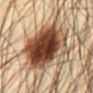Clinical summary: About 7 mm across. The tile uses cross-polarized illumination. A male patient in their mid- to late 40s. On the abdomen. A 15 mm close-up tile from a total-body photography series done for melanoma screening. Conclusion: Histopathologically confirmed as an atypical melanocytic neoplasm, classified as a lesion of indeterminate malignant potential.